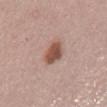Q: Was a biopsy performed?
A: imaged on a skin check; not biopsied
Q: Who is the patient?
A: male, aged approximately 75
Q: Lesion location?
A: the lower back
Q: What kind of image is this?
A: 15 mm crop, total-body photography
Q: Illumination type?
A: white-light
Q: What did automated image analysis measure?
A: an outline eccentricity of about 0.6 (0 = round, 1 = elongated) and a symmetry-axis asymmetry near 0.25; a border-irregularity index near 2/10, a color-variation rating of about 3/10, and peripheral color asymmetry of about 1
Q: What is the lesion's diameter?
A: about 3 mm